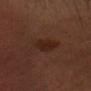Findings:
• biopsy status · no biopsy performed (imaged during a skin exam)
• tile lighting · cross-polarized illumination
• automated metrics · a footprint of about 6 mm², an outline eccentricity of about 0.75 (0 = round, 1 = elongated), and a symmetry-axis asymmetry near 0.15
• image source · ~15 mm crop, total-body skin-cancer survey
• lesion diameter · about 3.5 mm
• body site · the head or neck
• subject · male, roughly 45 years of age